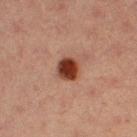The lesion was tiled from a total-body skin photograph and was not biopsied. This is a cross-polarized tile. A female subject, aged around 40. About 2.5 mm across. An algorithmic analysis of the crop reported a lesion-to-skin contrast of about 13.5 (normalized; higher = more distinct). And it measured a border-irregularity index near 1/10, a within-lesion color-variation index near 4/10, and a peripheral color-asymmetry measure near 1. The analysis additionally found a classifier nevus-likeness of about 100/100. A region of skin cropped from a whole-body photographic capture, roughly 15 mm wide. The lesion is located on the leg.